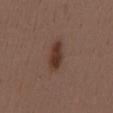The lesion is located on the mid back. The patient is a male in their 40s. A roughly 15 mm field-of-view crop from a total-body skin photograph. Automated image analysis of the tile measured an average lesion color of about L≈35 a*≈18 b*≈24 (CIELAB), roughly 10 lightness units darker than nearby skin, and a normalized lesion–skin contrast near 9. And it measured a nevus-likeness score of about 100/100 and a lesion-detection confidence of about 100/100.